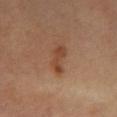No biopsy was performed on this lesion — it was imaged during a full skin examination and was not determined to be concerning.
The patient is a female aged around 65.
The tile uses cross-polarized illumination.
Cropped from a whole-body photographic skin survey; the tile spans about 15 mm.
On the right leg.
Automated image analysis of the tile measured a border-irregularity rating of about 4.5/10, internal color variation of about 1.5 on a 0–10 scale, and peripheral color asymmetry of about 0.5. The analysis additionally found an automated nevus-likeness rating near 30 out of 100 and lesion-presence confidence of about 100/100.